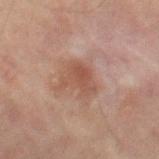Impression: No biopsy was performed on this lesion — it was imaged during a full skin examination and was not determined to be concerning. Image and clinical context: A lesion tile, about 15 mm wide, cut from a 3D total-body photograph. Imaged with cross-polarized lighting. The total-body-photography lesion software estimated an area of roughly 8.5 mm² and a shape eccentricity near 0.65. It also reported an automated nevus-likeness rating near 25 out of 100 and lesion-presence confidence of about 100/100. From the right thigh. Approximately 4 mm at its widest. A male patient, in their mid- to late 70s.